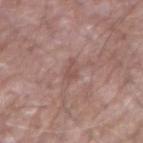Part of a total-body skin-imaging series; this lesion was reviewed on a skin check and was not flagged for biopsy.